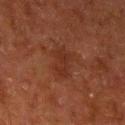follow-up: catalogued during a skin exam; not biopsied
subject: male, about 80 years old
location: the left lower leg
image: ~15 mm tile from a whole-body skin photo